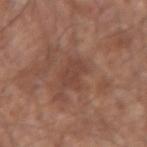Clinical impression: This lesion was catalogued during total-body skin photography and was not selected for biopsy. Context: Imaged with white-light lighting. A lesion tile, about 15 mm wide, cut from a 3D total-body photograph. The subject is a male in their mid-60s. Located on the left upper arm. The lesion-visualizer software estimated a lesion color around L≈43 a*≈21 b*≈26 in CIELAB, about 6 CIELAB-L* units darker than the surrounding skin, and a normalized border contrast of about 5. The recorded lesion diameter is about 3.5 mm.